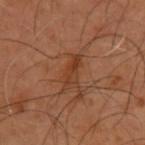workup = catalogued during a skin exam; not biopsied | patient = male, in their mid-50s | acquisition = ~15 mm tile from a whole-body skin photo | lesion size = ~4 mm (longest diameter) | location = the back | lighting = cross-polarized illumination | TBP lesion metrics = an average lesion color of about L≈37 a*≈23 b*≈32 (CIELAB), a lesion–skin lightness drop of about 7, and a normalized lesion–skin contrast near 6.5.